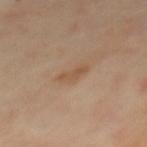notes: catalogued during a skin exam; not biopsied
image source: total-body-photography crop, ~15 mm field of view
diameter: ~3 mm (longest diameter)
patient: female, aged around 60
automated metrics: an average lesion color of about L≈53 a*≈19 b*≈32 (CIELAB) and a lesion–skin lightness drop of about 7; a within-lesion color-variation index near 0/10 and peripheral color asymmetry of about 0; a classifier nevus-likeness of about 5/100 and lesion-presence confidence of about 100/100
anatomic site: the back